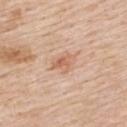Imaged during a routine full-body skin examination; the lesion was not biopsied and no histopathology is available. This is a white-light tile. The patient is a female aged approximately 70. The recorded lesion diameter is about 3 mm. Located on the upper back. A 15 mm close-up extracted from a 3D total-body photography capture.